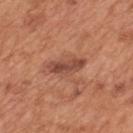Assessment: The lesion was photographed on a routine skin check and not biopsied; there is no pathology result. Background: Cropped from a total-body skin-imaging series; the visible field is about 15 mm. Longest diameter approximately 4.5 mm. Captured under white-light illumination. Located on the mid back. A male subject, roughly 65 years of age. The lesion-visualizer software estimated a footprint of about 6 mm², a shape eccentricity near 0.95, and two-axis asymmetry of about 0.25. And it measured a border-irregularity rating of about 3/10 and internal color variation of about 4 on a 0–10 scale.